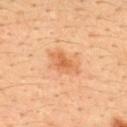Assessment: No biopsy was performed on this lesion — it was imaged during a full skin examination and was not determined to be concerning.